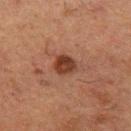  biopsy_status: not biopsied; imaged during a skin examination
  image:
    source: total-body photography crop
    field_of_view_mm: 15
  site: left upper arm
  patient:
    sex: male
    age_approx: 50